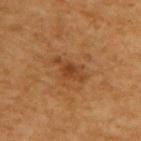Assessment: Part of a total-body skin-imaging series; this lesion was reviewed on a skin check and was not flagged for biopsy. Acquisition and patient details: Automated image analysis of the tile measured an area of roughly 5.5 mm², an outline eccentricity of about 0.9 (0 = round, 1 = elongated), and two-axis asymmetry of about 0.35. The analysis additionally found an average lesion color of about L≈39 a*≈21 b*≈35 (CIELAB), roughly 8 lightness units darker than nearby skin, and a lesion-to-skin contrast of about 6.5 (normalized; higher = more distinct). The lesion's longest dimension is about 4 mm. Captured under cross-polarized illumination. The patient is a male about 60 years old. This image is a 15 mm lesion crop taken from a total-body photograph. The lesion is located on the upper back.